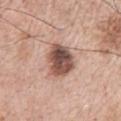Image and clinical context: A region of skin cropped from a whole-body photographic capture, roughly 15 mm wide. Located on the right upper arm. The lesion-visualizer software estimated an average lesion color of about L≈52 a*≈20 b*≈25 (CIELAB), about 18 CIELAB-L* units darker than the surrounding skin, and a lesion-to-skin contrast of about 11.5 (normalized; higher = more distinct). The software also gave a border-irregularity rating of about 2.5/10, a color-variation rating of about 8/10, and radial color variation of about 2.5. The software also gave a nevus-likeness score of about 55/100 and a lesion-detection confidence of about 100/100. The subject is a male in their mid- to late 60s.